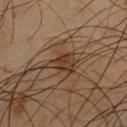This is a cross-polarized tile.
Measured at roughly 3.5 mm in maximum diameter.
Cropped from a whole-body photographic skin survey; the tile spans about 15 mm.
From the front of the torso.
A male subject aged 43 to 47.